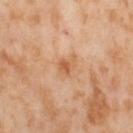The lesion was photographed on a routine skin check and not biopsied; there is no pathology result.
The lesion is on the left thigh.
The subject is a female in their mid-50s.
A 15 mm close-up tile from a total-body photography series done for melanoma screening.